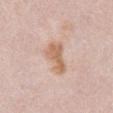Impression: This lesion was catalogued during total-body skin photography and was not selected for biopsy. Clinical summary: The lesion is on the front of the torso. The total-body-photography lesion software estimated a lesion area of about 9 mm² and a shape eccentricity near 0.9. And it measured a border-irregularity index near 3.5/10, a within-lesion color-variation index near 2.5/10, and peripheral color asymmetry of about 1. It also reported a classifier nevus-likeness of about 5/100. A female patient, in their mid-60s. This image is a 15 mm lesion crop taken from a total-body photograph. About 5 mm across.